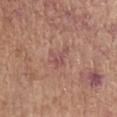Assessment: No biopsy was performed on this lesion — it was imaged during a full skin examination and was not determined to be concerning. Background: The recorded lesion diameter is about 3.5 mm. The tile uses white-light illumination. A lesion tile, about 15 mm wide, cut from a 3D total-body photograph. The lesion-visualizer software estimated internal color variation of about 2.5 on a 0–10 scale. Located on the left forearm. A female subject aged 63 to 67.